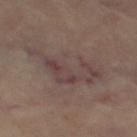Part of a total-body skin-imaging series; this lesion was reviewed on a skin check and was not flagged for biopsy.
This is a cross-polarized tile.
The lesion is on the left thigh.
Longest diameter approximately 8 mm.
Cropped from a total-body skin-imaging series; the visible field is about 15 mm.
The patient is a female aged 63 to 67.